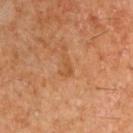Captured during whole-body skin photography for melanoma surveillance; the lesion was not biopsied. A 15 mm crop from a total-body photograph taken for skin-cancer surveillance. From the arm. Measured at roughly 3 mm in maximum diameter. Imaged with cross-polarized lighting. The subject is a male roughly 60 years of age.